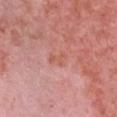Assessment: Part of a total-body skin-imaging series; this lesion was reviewed on a skin check and was not flagged for biopsy. Context: About 2.5 mm across. An algorithmic analysis of the crop reported an area of roughly 3 mm², an eccentricity of roughly 0.85, and a shape-asymmetry score of about 0.3 (0 = symmetric). The software also gave border irregularity of about 3.5 on a 0–10 scale, a within-lesion color-variation index near 0/10, and peripheral color asymmetry of about 0. A female patient, aged around 40. From the chest. A 15 mm close-up extracted from a 3D total-body photography capture. This is a white-light tile.